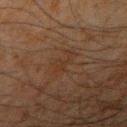workup: catalogued during a skin exam; not biopsied | illumination: cross-polarized | anatomic site: the arm | image: total-body-photography crop, ~15 mm field of view | subject: male, in their mid-30s | lesion size: about 3 mm.